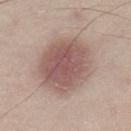{"biopsy_status": "not biopsied; imaged during a skin examination", "image": {"source": "total-body photography crop", "field_of_view_mm": 15}, "lesion_size": {"long_diameter_mm_approx": 7.0}, "patient": {"sex": "male", "age_approx": 45}, "site": "left lower leg", "lighting": "white-light"}